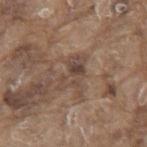notes = catalogued during a skin exam; not biopsied
lesion size = ≈4.5 mm
image = ~15 mm tile from a whole-body skin photo
illumination = white-light illumination
patient = male, aged 78 to 82
anatomic site = the mid back